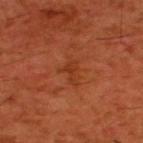The lesion was tiled from a total-body skin photograph and was not biopsied. From the upper back. Measured at roughly 2.5 mm in maximum diameter. The subject is a male aged around 60. A 15 mm crop from a total-body photograph taken for skin-cancer surveillance. This is a cross-polarized tile.